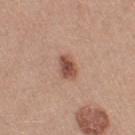<lesion>
<biopsy_status>not biopsied; imaged during a skin examination</biopsy_status>
<patient>
  <sex>female</sex>
  <age_approx>55</age_approx>
</patient>
<image>
  <source>total-body photography crop</source>
  <field_of_view_mm>15</field_of_view_mm>
</image>
<site>left thigh</site>
<lesion_size>
  <long_diameter_mm_approx>3.0</long_diameter_mm_approx>
</lesion_size>
<lighting>white-light</lighting>
<automated_metrics>
  <cielab_L>51</cielab_L>
  <cielab_a>22</cielab_a>
  <cielab_b>27</cielab_b>
  <vs_skin_darker_L>13.0</vs_skin_darker_L>
  <border_irregularity_0_10>2.0</border_irregularity_0_10>
  <color_variation_0_10>4.0</color_variation_0_10>
  <peripheral_color_asymmetry>1.0</peripheral_color_asymmetry>
  <nevus_likeness_0_100>95</nevus_likeness_0_100>
</automated_metrics>
</lesion>